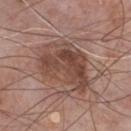Imaged during a routine full-body skin examination; the lesion was not biopsied and no histopathology is available.
The recorded lesion diameter is about 7 mm.
This is a white-light tile.
A male subject, aged around 75.
A close-up tile cropped from a whole-body skin photograph, about 15 mm across.
The lesion-visualizer software estimated a footprint of about 25 mm², an outline eccentricity of about 0.75 (0 = round, 1 = elongated), and two-axis asymmetry of about 0.3. The analysis additionally found an average lesion color of about L≈45 a*≈19 b*≈25 (CIELAB), roughly 10 lightness units darker than nearby skin, and a lesion-to-skin contrast of about 8 (normalized; higher = more distinct). And it measured a nevus-likeness score of about 55/100.
On the chest.